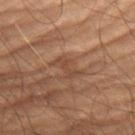Assessment:
Recorded during total-body skin imaging; not selected for excision or biopsy.
Background:
Located on the left thigh. A male patient roughly 70 years of age. The lesion's longest dimension is about 3 mm. Automated image analysis of the tile measured an outline eccentricity of about 0.85 (0 = round, 1 = elongated) and a symmetry-axis asymmetry near 0.35. And it measured a mean CIELAB color near L≈33 a*≈16 b*≈23. It also reported a border-irregularity rating of about 4/10 and internal color variation of about 0.5 on a 0–10 scale. This is a cross-polarized tile. A roughly 15 mm field-of-view crop from a total-body skin photograph.